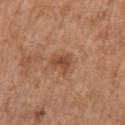• workup · imaged on a skin check; not biopsied
• lesion diameter · about 2.5 mm
• subject · female, roughly 75 years of age
• image source · ~15 mm tile from a whole-body skin photo
• anatomic site · the right upper arm